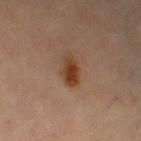Assessment:
Part of a total-body skin-imaging series; this lesion was reviewed on a skin check and was not flagged for biopsy.
Context:
Imaged with cross-polarized lighting. The total-body-photography lesion software estimated a lesion area of about 6.5 mm² and an eccentricity of roughly 0.8. The analysis additionally found a border-irregularity rating of about 2/10, a color-variation rating of about 5.5/10, and a peripheral color-asymmetry measure near 1.5. And it measured an automated nevus-likeness rating near 100 out of 100 and a lesion-detection confidence of about 100/100. Cropped from a total-body skin-imaging series; the visible field is about 15 mm. About 3.5 mm across. The lesion is on the left thigh. A female patient in their 70s.